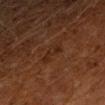Q: Was a biopsy performed?
A: imaged on a skin check; not biopsied
Q: What kind of image is this?
A: 15 mm crop, total-body photography
Q: What is the lesion's diameter?
A: ≈3 mm
Q: Illumination type?
A: cross-polarized
Q: What are the patient's age and sex?
A: male, aged approximately 60
Q: Where on the body is the lesion?
A: the left forearm